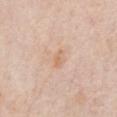Imaged during a routine full-body skin examination; the lesion was not biopsied and no histopathology is available.
A roughly 15 mm field-of-view crop from a total-body skin photograph.
The lesion is located on the chest.
Measured at roughly 2.5 mm in maximum diameter.
Captured under white-light illumination.
A male patient, aged approximately 75.
Automated image analysis of the tile measured a lesion color around L≈67 a*≈19 b*≈33 in CIELAB and a lesion–skin lightness drop of about 7. The analysis additionally found a border-irregularity rating of about 2.5/10, a within-lesion color-variation index near 1/10, and a peripheral color-asymmetry measure near 0.5. And it measured an automated nevus-likeness rating near 0 out of 100.